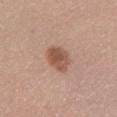follow-up: total-body-photography surveillance lesion; no biopsy | image: total-body-photography crop, ~15 mm field of view | subject: female, in their mid- to late 30s | location: the left lower leg | diameter: about 3.5 mm | tile lighting: white-light illumination.